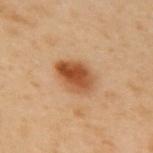No biopsy was performed on this lesion — it was imaged during a full skin examination and was not determined to be concerning. A female patient aged 58–62. Located on the upper back. Measured at roughly 4 mm in maximum diameter. A 15 mm crop from a total-body photograph taken for skin-cancer surveillance. Captured under cross-polarized illumination.